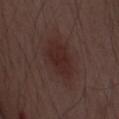Impression: Part of a total-body skin-imaging series; this lesion was reviewed on a skin check and was not flagged for biopsy. Context: On the chest. A 15 mm close-up tile from a total-body photography series done for melanoma screening. A male patient, aged approximately 55. Approximately 5.5 mm at its widest. This is a white-light tile.